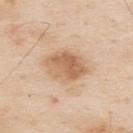Recorded during total-body skin imaging; not selected for excision or biopsy. The subject is a male roughly 80 years of age. A region of skin cropped from a whole-body photographic capture, roughly 15 mm wide. The lesion is on the upper back.